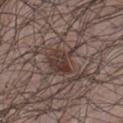follow-up: total-body-photography surveillance lesion; no biopsy | lesion size: about 3.5 mm | site: the chest | lighting: white-light | image: ~15 mm crop, total-body skin-cancer survey | patient: male, approximately 40 years of age.